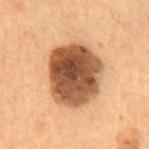Q: Was a biopsy performed?
A: no biopsy performed (imaged during a skin exam)
Q: Where on the body is the lesion?
A: the abdomen
Q: What kind of image is this?
A: total-body-photography crop, ~15 mm field of view
Q: What is the lesion's diameter?
A: ≈7 mm
Q: How was the tile lit?
A: cross-polarized illumination
Q: What are the patient's age and sex?
A: male, roughly 55 years of age